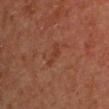Cropped from a total-body skin-imaging series; the visible field is about 15 mm.
From the right upper arm.
The tile uses cross-polarized illumination.
The recorded lesion diameter is about 3 mm.
The patient is a male aged approximately 65.